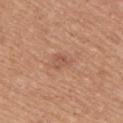Part of a total-body skin-imaging series; this lesion was reviewed on a skin check and was not flagged for biopsy. From the right upper arm. Approximately 2.5 mm at its widest. A female patient aged 43–47. A 15 mm close-up tile from a total-body photography series done for melanoma screening.